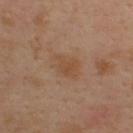  biopsy_status: not biopsied; imaged during a skin examination
  automated_metrics:
    nevus_likeness_0_100: 5
    lesion_detection_confidence_0_100: 100
  site: back
  lesion_size:
    long_diameter_mm_approx: 3.5
  image:
    source: total-body photography crop
    field_of_view_mm: 15
  lighting: cross-polarized
  patient:
    sex: male
    age_approx: 35